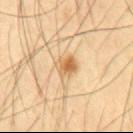Captured during whole-body skin photography for melanoma surveillance; the lesion was not biopsied.
A 15 mm close-up tile from a total-body photography series done for melanoma screening.
Located on the abdomen.
The tile uses cross-polarized illumination.
A male patient aged 58–62.
An algorithmic analysis of the crop reported an area of roughly 5.5 mm² and a shape eccentricity near 0.65. And it measured roughly 12 lightness units darker than nearby skin and a lesion-to-skin contrast of about 8 (normalized; higher = more distinct).